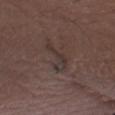Assessment: Captured during whole-body skin photography for melanoma surveillance; the lesion was not biopsied. Acquisition and patient details: A 15 mm close-up tile from a total-body photography series done for melanoma screening. The patient is a male aged around 50. The lesion is located on the left forearm.